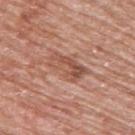The patient is a female roughly 60 years of age. A close-up tile cropped from a whole-body skin photograph, about 15 mm across. The lesion is on the upper back.